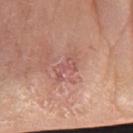notes=catalogued during a skin exam; not biopsied | diameter=about 4 mm | subject=female, approximately 75 years of age | anatomic site=the right forearm | lighting=white-light illumination | acquisition=~15 mm tile from a whole-body skin photo.